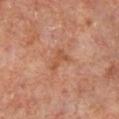Findings:
- notes — no biopsy performed (imaged during a skin exam)
- body site — the leg
- tile lighting — cross-polarized illumination
- subject — male, in their mid- to late 60s
- image source — 15 mm crop, total-body photography